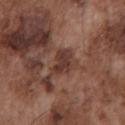notes: imaged on a skin check; not biopsied
image source: total-body-photography crop, ~15 mm field of view
lesion size: ~3.5 mm (longest diameter)
patient: male, aged 73 to 77
lighting: white-light illumination
image-analysis metrics: a footprint of about 6 mm²; a mean CIELAB color near L≈36 a*≈20 b*≈23, roughly 10 lightness units darker than nearby skin, and a normalized lesion–skin contrast near 8.5; a border-irregularity rating of about 3.5/10, a within-lesion color-variation index near 2.5/10, and a peripheral color-asymmetry measure near 1
anatomic site: the chest